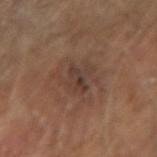Case summary:
- image source: ~15 mm tile from a whole-body skin photo
- tile lighting: cross-polarized illumination
- subject: male, in their mid- to late 60s
- site: the arm
- lesion diameter: about 5 mm